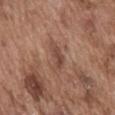  biopsy_status: not biopsied; imaged during a skin examination
  patient:
    sex: male
    age_approx: 75
  image:
    source: total-body photography crop
    field_of_view_mm: 15
  site: abdomen
  lesion_size:
    long_diameter_mm_approx: 4.0
  lighting: white-light